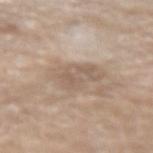From the right forearm.
Imaged with white-light lighting.
The subject is a male roughly 70 years of age.
Approximately 5 mm at its widest.
Cropped from a whole-body photographic skin survey; the tile spans about 15 mm.
Automated image analysis of the tile measured a border-irregularity rating of about 3/10. And it measured a nevus-likeness score of about 0/100 and a detector confidence of about 60 out of 100 that the crop contains a lesion.